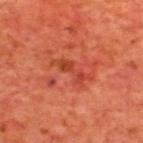This lesion was catalogued during total-body skin photography and was not selected for biopsy. From the upper back. Cropped from a total-body skin-imaging series; the visible field is about 15 mm. A male patient, in their mid- to late 60s.